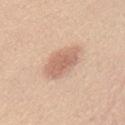<case>
  <biopsy_status>not biopsied; imaged during a skin examination</biopsy_status>
  <lesion_size>
    <long_diameter_mm_approx>5.0</long_diameter_mm_approx>
  </lesion_size>
  <site>chest</site>
  <patient>
    <sex>female</sex>
    <age_approx>40</age_approx>
  </patient>
  <image>
    <source>total-body photography crop</source>
    <field_of_view_mm>15</field_of_view_mm>
  </image>
  <lighting>white-light</lighting>
</case>